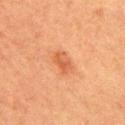– size · about 3 mm
– illumination · cross-polarized
– subject · female, aged 53–57
– automated lesion analysis · a lesion color around L≈49 a*≈25 b*≈35 in CIELAB, about 7 CIELAB-L* units darker than the surrounding skin, and a normalized lesion–skin contrast near 6; a nevus-likeness score of about 70/100 and lesion-presence confidence of about 100/100
– anatomic site · the right upper arm
– image source · 15 mm crop, total-body photography